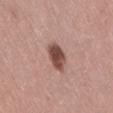Part of a total-body skin-imaging series; this lesion was reviewed on a skin check and was not flagged for biopsy. From the lower back. A female patient, approximately 40 years of age. The recorded lesion diameter is about 3.5 mm. Cropped from a whole-body photographic skin survey; the tile spans about 15 mm.